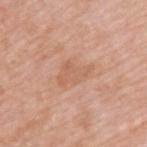Recorded during total-body skin imaging; not selected for excision or biopsy. Longest diameter approximately 4 mm. Located on the upper back. A lesion tile, about 15 mm wide, cut from a 3D total-body photograph. Imaged with white-light lighting. The subject is a male about 65 years old.